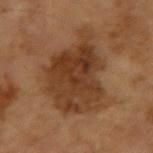Q: Is there a histopathology result?
A: imaged on a skin check; not biopsied
Q: What kind of image is this?
A: ~15 mm tile from a whole-body skin photo
Q: Automated lesion metrics?
A: an average lesion color of about L≈36 a*≈20 b*≈31 (CIELAB) and about 10 CIELAB-L* units darker than the surrounding skin
Q: Patient demographics?
A: male, roughly 65 years of age
Q: What is the anatomic site?
A: the left arm
Q: Lesion size?
A: ~8.5 mm (longest diameter)